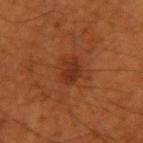Q: Was a biopsy performed?
A: imaged on a skin check; not biopsied
Q: Who is the patient?
A: male, approximately 55 years of age
Q: Lesion size?
A: about 3 mm
Q: What is the anatomic site?
A: the right upper arm
Q: Automated lesion metrics?
A: an area of roughly 5 mm² and an eccentricity of roughly 0.65; a classifier nevus-likeness of about 50/100 and a lesion-detection confidence of about 100/100
Q: What lighting was used for the tile?
A: cross-polarized illumination
Q: What kind of image is this?
A: ~15 mm crop, total-body skin-cancer survey